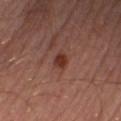Findings:
* location — the right lower leg
* imaging modality — total-body-photography crop, ~15 mm field of view
* subject — male, aged approximately 55
* image-analysis metrics — border irregularity of about 2 on a 0–10 scale; an automated nevus-likeness rating near 85 out of 100 and a detector confidence of about 100 out of 100 that the crop contains a lesion
* lighting — cross-polarized